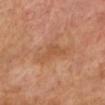The lesion was photographed on a routine skin check and not biopsied; there is no pathology result.
About 4 mm across.
A female subject about 55 years old.
The lesion is on the left lower leg.
A 15 mm close-up tile from a total-body photography series done for melanoma screening.
The tile uses cross-polarized illumination.
Automated image analysis of the tile measured a lesion area of about 7 mm² and two-axis asymmetry of about 0.55.